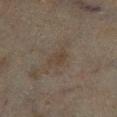workup=no biopsy performed (imaged during a skin exam) | location=the leg | lesion size=~2.5 mm (longest diameter) | patient=male, about 70 years old | image=total-body-photography crop, ~15 mm field of view.